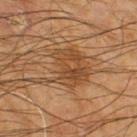• biopsy status · catalogued during a skin exam; not biopsied
• location · the left thigh
• automated metrics · a footprint of about 17 mm², an outline eccentricity of about 0.55 (0 = round, 1 = elongated), and a shape-asymmetry score of about 0.45 (0 = symmetric); about 7 CIELAB-L* units darker than the surrounding skin and a lesion-to-skin contrast of about 6.5 (normalized; higher = more distinct); a classifier nevus-likeness of about 40/100 and a detector confidence of about 100 out of 100 that the crop contains a lesion
• tile lighting · cross-polarized
• imaging modality · 15 mm crop, total-body photography
• subject · male, aged around 65